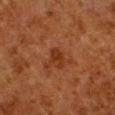Q: Was this lesion biopsied?
A: total-body-photography surveillance lesion; no biopsy
Q: Where on the body is the lesion?
A: the left lower leg
Q: Patient demographics?
A: male, aged approximately 80
Q: How large is the lesion?
A: ~2.5 mm (longest diameter)
Q: What is the imaging modality?
A: ~15 mm crop, total-body skin-cancer survey
Q: What lighting was used for the tile?
A: cross-polarized illumination
Q: Automated lesion metrics?
A: a mean CIELAB color near L≈26 a*≈22 b*≈29, about 6 CIELAB-L* units darker than the surrounding skin, and a lesion-to-skin contrast of about 7 (normalized; higher = more distinct); a detector confidence of about 100 out of 100 that the crop contains a lesion